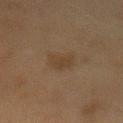Assessment:
This lesion was catalogued during total-body skin photography and was not selected for biopsy.
Clinical summary:
Cropped from a whole-body photographic skin survey; the tile spans about 15 mm. A female subject aged around 50. Captured under cross-polarized illumination. Approximately 3 mm at its widest. The lesion is located on the chest.